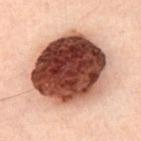About 8.5 mm across.
The patient is a male about 50 years old.
From the chest.
Captured under cross-polarized illumination.
A 15 mm close-up extracted from a 3D total-body photography capture.